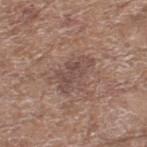Imaged during a routine full-body skin examination; the lesion was not biopsied and no histopathology is available. Cropped from a total-body skin-imaging series; the visible field is about 15 mm. A female patient, in their mid- to late 70s. Approximately 4.5 mm at its widest. This is a white-light tile. Located on the leg.